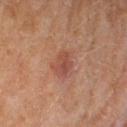<tbp_lesion>
  <biopsy_status>not biopsied; imaged during a skin examination</biopsy_status>
</tbp_lesion>